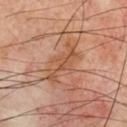  automated_metrics:
    area_mm2_approx: 7.0
    eccentricity: 0.9
    shape_asymmetry: 0.5
    peripheral_color_asymmetry: 0.5
    nevus_likeness_0_100: 0
    lesion_detection_confidence_0_100: 95
  site: chest
  image:
    source: total-body photography crop
    field_of_view_mm: 15
  lesion_size:
    long_diameter_mm_approx: 5.0
  patient:
    sex: male
    age_approx: 70
  lighting: cross-polarized